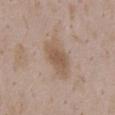follow-up = no biopsy performed (imaged during a skin exam) | lighting = white-light | automated metrics = a classifier nevus-likeness of about 35/100 | patient = female, aged 33 to 37 | image source = 15 mm crop, total-body photography | location = the chest | size = ≈5 mm.